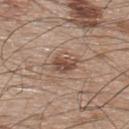Recorded during total-body skin imaging; not selected for excision or biopsy.
The lesion is on the upper back.
The lesion-visualizer software estimated an area of roughly 4.5 mm² and an eccentricity of roughly 0.7. And it measured a lesion color around L≈48 a*≈18 b*≈27 in CIELAB, roughly 11 lightness units darker than nearby skin, and a normalized border contrast of about 8.5. The analysis additionally found a border-irregularity index near 3/10, a color-variation rating of about 4.5/10, and a peripheral color-asymmetry measure near 2. It also reported a detector confidence of about 100 out of 100 that the crop contains a lesion.
The patient is a male aged approximately 50.
Cropped from a total-body skin-imaging series; the visible field is about 15 mm.